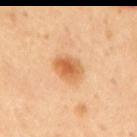biopsy status: catalogued during a skin exam; not biopsied | subject: male, approximately 65 years of age | lighting: cross-polarized | diameter: about 3.5 mm | image source: 15 mm crop, total-body photography | image-analysis metrics: a lesion area of about 8 mm², an eccentricity of roughly 0.75, and a symmetry-axis asymmetry near 0.15 | site: the mid back.